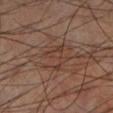No biopsy was performed on this lesion — it was imaged during a full skin examination and was not determined to be concerning. Located on the leg. A region of skin cropped from a whole-body photographic capture, roughly 15 mm wide. The patient is a male aged around 60.